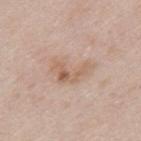biopsy status: imaged on a skin check; not biopsied | image source: total-body-photography crop, ~15 mm field of view | location: the back | patient: male, approximately 40 years of age | lighting: white-light | lesion diameter: about 5.5 mm.